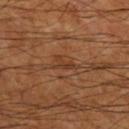Impression: No biopsy was performed on this lesion — it was imaged during a full skin examination and was not determined to be concerning. Context: Cropped from a whole-body photographic skin survey; the tile spans about 15 mm. The total-body-photography lesion software estimated a lesion color around L≈37 a*≈21 b*≈32 in CIELAB, about 7 CIELAB-L* units darker than the surrounding skin, and a normalized border contrast of about 6. The tile uses cross-polarized illumination. Located on the leg. The subject is a male aged approximately 60.